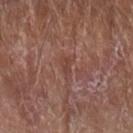Impression: Part of a total-body skin-imaging series; this lesion was reviewed on a skin check and was not flagged for biopsy. Background: The recorded lesion diameter is about 2.5 mm. The lesion is located on the arm. Automated tile analysis of the lesion measured a border-irregularity index near 3.5/10 and peripheral color asymmetry of about 0. A male patient, aged around 85 or older. This is a white-light tile. This image is a 15 mm lesion crop taken from a total-body photograph.